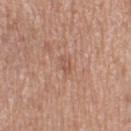This lesion was catalogued during total-body skin photography and was not selected for biopsy. Located on the left thigh. The patient is a female in their 40s. A roughly 15 mm field-of-view crop from a total-body skin photograph. The recorded lesion diameter is about 2.5 mm.